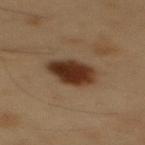The lesion was tiled from a total-body skin photograph and was not biopsied.
A roughly 15 mm field-of-view crop from a total-body skin photograph.
Located on the mid back.
The patient is a male about 55 years old.